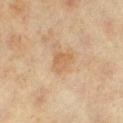Imaged during a routine full-body skin examination; the lesion was not biopsied and no histopathology is available.
Cropped from a whole-body photographic skin survey; the tile spans about 15 mm.
On the left lower leg.
The patient is a female aged around 40.
The recorded lesion diameter is about 2.5 mm.
Imaged with cross-polarized lighting.